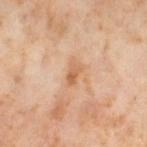This lesion was catalogued during total-body skin photography and was not selected for biopsy.
Captured under cross-polarized illumination.
The lesion's longest dimension is about 3 mm.
A roughly 15 mm field-of-view crop from a total-body skin photograph.
On the right thigh.
A female patient, in their mid- to late 50s.